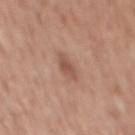Assessment:
This lesion was catalogued during total-body skin photography and was not selected for biopsy.
Background:
The recorded lesion diameter is about 2.5 mm. An algorithmic analysis of the crop reported a footprint of about 3.5 mm², an outline eccentricity of about 0.8 (0 = round, 1 = elongated), and a shape-asymmetry score of about 0.3 (0 = symmetric). It also reported an average lesion color of about L≈52 a*≈21 b*≈28 (CIELAB) and a normalized border contrast of about 7. A 15 mm close-up extracted from a 3D total-body photography capture. A male subject, aged around 60. On the back.